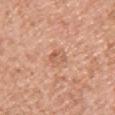  biopsy_status: not biopsied; imaged during a skin examination
  site: chest
  image:
    source: total-body photography crop
    field_of_view_mm: 15
  lighting: white-light
  automated_metrics:
    area_mm2_approx: 4.0
    eccentricity: 0.8
    shape_asymmetry: 0.2
    border_irregularity_0_10: 2.0
    color_variation_0_10: 3.0
    peripheral_color_asymmetry: 1.0
    nevus_likeness_0_100: 0
    lesion_detection_confidence_0_100: 100
  patient:
    sex: female
    age_approx: 40